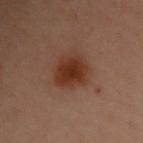Captured under cross-polarized illumination. A female patient aged 58 to 62. The lesion's longest dimension is about 4.5 mm. A roughly 15 mm field-of-view crop from a total-body skin photograph. The lesion is located on the back.